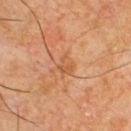Impression: Imaged during a routine full-body skin examination; the lesion was not biopsied and no histopathology is available. Context: A 15 mm close-up tile from a total-body photography series done for melanoma screening. The recorded lesion diameter is about 2.5 mm. A male patient aged approximately 65. On the chest.